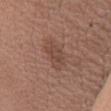No biopsy was performed on this lesion — it was imaged during a full skin examination and was not determined to be concerning.
On the chest.
The recorded lesion diameter is about 3.5 mm.
A female patient, roughly 50 years of age.
A close-up tile cropped from a whole-body skin photograph, about 15 mm across.